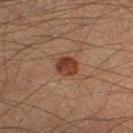<case>
  <biopsy_status>not biopsied; imaged during a skin examination</biopsy_status>
  <patient>
    <sex>male</sex>
    <age_approx>40</age_approx>
  </patient>
  <image>
    <source>total-body photography crop</source>
    <field_of_view_mm>15</field_of_view_mm>
  </image>
  <automated_metrics>
    <eccentricity>0.55</eccentricity>
    <shape_asymmetry>0.15</shape_asymmetry>
    <cielab_L>34</cielab_L>
    <cielab_a>21</cielab_a>
    <cielab_b>27</cielab_b>
    <vs_skin_darker_L>11.0</vs_skin_darker_L>
    <vs_skin_contrast_norm>10.0</vs_skin_contrast_norm>
  </automated_metrics>
  <site>left lower leg</site>
  <lesion_size>
    <long_diameter_mm_approx>2.5</long_diameter_mm_approx>
  </lesion_size>
  <lighting>cross-polarized</lighting>
</case>